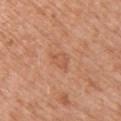Part of a total-body skin-imaging series; this lesion was reviewed on a skin check and was not flagged for biopsy.
A close-up tile cropped from a whole-body skin photograph, about 15 mm across.
Located on the right upper arm.
The patient is a female in their 40s.
The tile uses white-light illumination.
About 3 mm across.